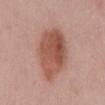No biopsy was performed on this lesion — it was imaged during a full skin examination and was not determined to be concerning.
The patient is a male roughly 55 years of age.
A 15 mm close-up extracted from a 3D total-body photography capture.
On the front of the torso.
Approximately 8 mm at its widest.
Captured under white-light illumination.